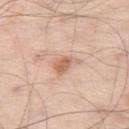Clinical impression:
Part of a total-body skin-imaging series; this lesion was reviewed on a skin check and was not flagged for biopsy.
Clinical summary:
The tile uses white-light illumination. On the left thigh. Approximately 3 mm at its widest. A male patient, aged around 55. Cropped from a whole-body photographic skin survey; the tile spans about 15 mm. Automated image analysis of the tile measured a footprint of about 4.5 mm², an eccentricity of roughly 0.8, and a symmetry-axis asymmetry near 0.35. It also reported a lesion color around L≈64 a*≈21 b*≈31 in CIELAB, roughly 11 lightness units darker than nearby skin, and a normalized lesion–skin contrast near 7. The software also gave a border-irregularity rating of about 4/10, a color-variation rating of about 2/10, and radial color variation of about 0.5.